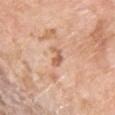Case summary:
• notes: total-body-photography surveillance lesion; no biopsy
• subject: male, aged 58 to 62
• imaging modality: 15 mm crop, total-body photography
• lesion size: ≈2.5 mm
• body site: the chest
• automated metrics: a footprint of about 3 mm² and an outline eccentricity of about 0.85 (0 = round, 1 = elongated); about 11 CIELAB-L* units darker than the surrounding skin and a normalized lesion–skin contrast near 7; a within-lesion color-variation index near 0/10
• tile lighting: white-light illumination